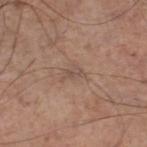{
  "biopsy_status": "not biopsied; imaged during a skin examination",
  "lesion_size": {
    "long_diameter_mm_approx": 2.5
  },
  "automated_metrics": {
    "area_mm2_approx": 2.5,
    "eccentricity": 0.85,
    "shape_asymmetry": 0.4,
    "border_irregularity_0_10": 5.0,
    "peripheral_color_asymmetry": 0.0,
    "nevus_likeness_0_100": 0,
    "lesion_detection_confidence_0_100": 95
  },
  "lighting": "white-light",
  "site": "right lower leg",
  "image": {
    "source": "total-body photography crop",
    "field_of_view_mm": 15
  },
  "patient": {
    "sex": "male",
    "age_approx": 60
  }
}